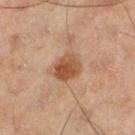Impression: The lesion was tiled from a total-body skin photograph and was not biopsied. Context: From the left lower leg. Captured under cross-polarized illumination. A region of skin cropped from a whole-body photographic capture, roughly 15 mm wide. A male patient roughly 45 years of age.